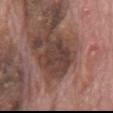- follow-up: total-body-photography surveillance lesion; no biopsy
- location: the mid back
- subject: male, about 70 years old
- acquisition: ~15 mm tile from a whole-body skin photo